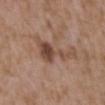Clinical impression: Part of a total-body skin-imaging series; this lesion was reviewed on a skin check and was not flagged for biopsy. Image and clinical context: The lesion's longest dimension is about 6 mm. The tile uses white-light illumination. A region of skin cropped from a whole-body photographic capture, roughly 15 mm wide. The lesion is on the upper back. A female patient, roughly 35 years of age.